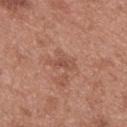| key | value |
|---|---|
| biopsy status | catalogued during a skin exam; not biopsied |
| lighting | white-light illumination |
| location | the back |
| image | ~15 mm crop, total-body skin-cancer survey |
| lesion diameter | ~3 mm (longest diameter) |
| patient | female, approximately 40 years of age |